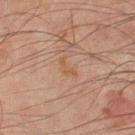Impression:
This lesion was catalogued during total-body skin photography and was not selected for biopsy.